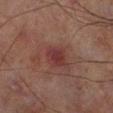Q: Is there a histopathology result?
A: no biopsy performed (imaged during a skin exam)
Q: Patient demographics?
A: male, approximately 70 years of age
Q: What is the imaging modality?
A: ~15 mm crop, total-body skin-cancer survey
Q: What did automated image analysis measure?
A: two-axis asymmetry of about 0.2; a lesion color around L≈28 a*≈20 b*≈18 in CIELAB, roughly 6 lightness units darker than nearby skin, and a lesion-to-skin contrast of about 7 (normalized; higher = more distinct)
Q: What is the anatomic site?
A: the leg
Q: How was the tile lit?
A: cross-polarized